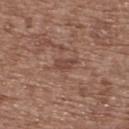The lesion was photographed on a routine skin check and not biopsied; there is no pathology result. On the back. This is a white-light tile. This image is a 15 mm lesion crop taken from a total-body photograph. The patient is a female aged 73 to 77. Automated tile analysis of the lesion measured a mean CIELAB color near L≈44 a*≈20 b*≈25 and a lesion-to-skin contrast of about 6.5 (normalized; higher = more distinct).